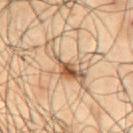Captured during whole-body skin photography for melanoma surveillance; the lesion was not biopsied. A male subject in their 50s. The lesion's longest dimension is about 6.5 mm. A 15 mm close-up extracted from a 3D total-body photography capture. The lesion is on the front of the torso.